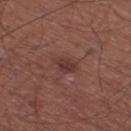Clinical impression:
Imaged during a routine full-body skin examination; the lesion was not biopsied and no histopathology is available.
Image and clinical context:
The subject is a male approximately 65 years of age. Longest diameter approximately 3 mm. Captured under white-light illumination. A region of skin cropped from a whole-body photographic capture, roughly 15 mm wide. The lesion is on the right thigh.